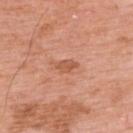Q: Was this lesion biopsied?
A: total-body-photography surveillance lesion; no biopsy
Q: Automated lesion metrics?
A: an outline eccentricity of about 0.85 (0 = round, 1 = elongated); a lesion color around L≈56 a*≈26 b*≈34 in CIELAB, roughly 9 lightness units darker than nearby skin, and a lesion-to-skin contrast of about 6.5 (normalized; higher = more distinct); an automated nevus-likeness rating near 0 out of 100 and a detector confidence of about 100 out of 100 that the crop contains a lesion
Q: How large is the lesion?
A: ~2.5 mm (longest diameter)
Q: What are the patient's age and sex?
A: male, aged 58–62
Q: How was this image acquired?
A: total-body-photography crop, ~15 mm field of view
Q: What is the anatomic site?
A: the upper back